A male patient, aged 73–77. Cropped from a total-body skin-imaging series; the visible field is about 15 mm. The lesion is located on the left forearm:
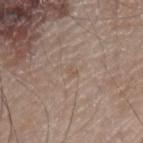Captured under white-light illumination.
The total-body-photography lesion software estimated a footprint of about 1.5 mm² and a shape-asymmetry score of about 0.35 (0 = symmetric). And it measured a mean CIELAB color near L≈54 a*≈14 b*≈26 and roughly 5 lightness units darker than nearby skin. The software also gave a border-irregularity index near 2.5/10 and a peripheral color-asymmetry measure near 0. And it measured a classifier nevus-likeness of about 0/100.
The lesion's longest dimension is about 1.5 mm.
Histopathology of the biopsied lesion showed a lichen planus-like keratosis, classified as a benign skin lesion.